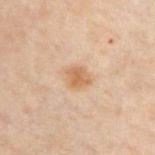{
  "biopsy_status": "not biopsied; imaged during a skin examination",
  "patient": {
    "sex": "male",
    "age_approx": 50
  },
  "image": {
    "source": "total-body photography crop",
    "field_of_view_mm": 15
  },
  "site": "chest"
}